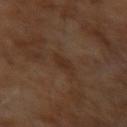  biopsy_status: not biopsied; imaged during a skin examination
  patient:
    sex: male
    age_approx: 65
  automated_metrics:
    area_mm2_approx: 4.0
    eccentricity: 0.75
    shape_asymmetry: 0.25
    border_irregularity_0_10: 3.0
    color_variation_0_10: 1.0
    peripheral_color_asymmetry: 0.5
    nevus_likeness_0_100: 0
    lesion_detection_confidence_0_100: 100
  lighting: cross-polarized
  image:
    source: total-body photography crop
    field_of_view_mm: 15
  lesion_size:
    long_diameter_mm_approx: 3.0